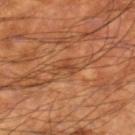follow-up: imaged on a skin check; not biopsied | body site: the right lower leg | lesion size: ~2.5 mm (longest diameter) | imaging modality: ~15 mm tile from a whole-body skin photo | subject: male, approximately 60 years of age | illumination: cross-polarized illumination | automated metrics: an outline eccentricity of about 0.8 (0 = round, 1 = elongated) and a symmetry-axis asymmetry near 0.55; a lesion–skin lightness drop of about 8 and a normalized border contrast of about 6; a nevus-likeness score of about 0/100 and lesion-presence confidence of about 90/100.